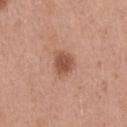Cropped from a whole-body photographic skin survey; the tile spans about 15 mm.
The subject is a female in their 40s.
The lesion's longest dimension is about 3 mm.
Captured under white-light illumination.
Located on the right thigh.